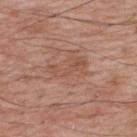notes: imaged on a skin check; not biopsied | anatomic site: the upper back | acquisition: ~15 mm tile from a whole-body skin photo | patient: male, roughly 70 years of age.